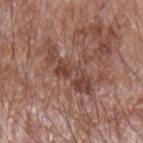{"biopsy_status": "not biopsied; imaged during a skin examination", "patient": {"sex": "male", "age_approx": 60}, "image": {"source": "total-body photography crop", "field_of_view_mm": 15}, "lesion_size": {"long_diameter_mm_approx": 7.5}, "site": "left forearm", "lighting": "white-light", "automated_metrics": {"nevus_likeness_0_100": 0, "lesion_detection_confidence_0_100": 85}}